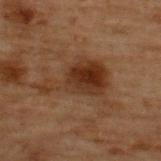Q: Is there a histopathology result?
A: total-body-photography surveillance lesion; no biopsy
Q: How was this image acquired?
A: ~15 mm crop, total-body skin-cancer survey
Q: Who is the patient?
A: male, in their 70s
Q: Automated lesion metrics?
A: a footprint of about 18 mm² and an outline eccentricity of about 0.8 (0 = round, 1 = elongated); a mean CIELAB color near L≈25 a*≈16 b*≈24, about 8 CIELAB-L* units darker than the surrounding skin, and a normalized border contrast of about 8.5; a within-lesion color-variation index near 5/10 and radial color variation of about 1.5
Q: How large is the lesion?
A: about 7 mm
Q: Illumination type?
A: cross-polarized
Q: Lesion location?
A: the upper back